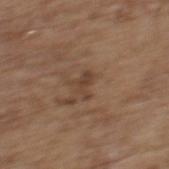Image and clinical context:
The patient is a female approximately 65 years of age. On the upper back. The lesion-visualizer software estimated an outline eccentricity of about 0.8 (0 = round, 1 = elongated). The analysis additionally found an average lesion color of about L≈41 a*≈17 b*≈27 (CIELAB), about 7 CIELAB-L* units darker than the surrounding skin, and a normalized lesion–skin contrast near 6.5. It also reported border irregularity of about 6.5 on a 0–10 scale, a color-variation rating of about 0.5/10, and peripheral color asymmetry of about 0. Longest diameter approximately 2.5 mm. Captured under white-light illumination. A lesion tile, about 15 mm wide, cut from a 3D total-body photograph.